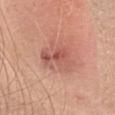- lesion diameter: ≈5 mm
- illumination: white-light illumination
- anatomic site: the head or neck
- subject: female, in their mid-20s
- image source: ~15 mm tile from a whole-body skin photo